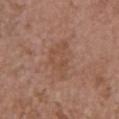{"patient": {"sex": "female", "age_approx": 65}, "image": {"source": "total-body photography crop", "field_of_view_mm": 15}, "automated_metrics": {"area_mm2_approx": 8.0, "border_irregularity_0_10": 6.5, "color_variation_0_10": 3.5, "peripheral_color_asymmetry": 1.0, "nevus_likeness_0_100": 0, "lesion_detection_confidence_0_100": 100}, "site": "chest", "lesion_size": {"long_diameter_mm_approx": 5.0}}